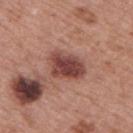notes = total-body-photography surveillance lesion; no biopsy
anatomic site = the upper back
lesion size = ~4.5 mm (longest diameter)
illumination = white-light illumination
automated lesion analysis = an area of roughly 11 mm² and a symmetry-axis asymmetry near 0.2; an average lesion color of about L≈44 a*≈25 b*≈24 (CIELAB), a lesion–skin lightness drop of about 14, and a normalized border contrast of about 10.5; a border-irregularity index near 2.5/10
image source = 15 mm crop, total-body photography
subject = male, aged 63 to 67